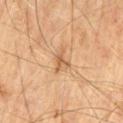The lesion was photographed on a routine skin check and not biopsied; there is no pathology result.
A close-up tile cropped from a whole-body skin photograph, about 15 mm across.
From the left thigh.
The patient is a male in their 70s.